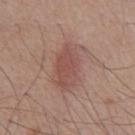Recorded during total-body skin imaging; not selected for excision or biopsy. Automated image analysis of the tile measured a mean CIELAB color near L≈51 a*≈21 b*≈24, a lesion–skin lightness drop of about 8, and a normalized border contrast of about 6. And it measured border irregularity of about 3 on a 0–10 scale, a color-variation rating of about 2.5/10, and radial color variation of about 1. Imaged with white-light lighting. A region of skin cropped from a whole-body photographic capture, roughly 15 mm wide. The recorded lesion diameter is about 6 mm. The subject is a male aged 73–77. Located on the chest.